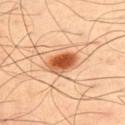lighting: cross-polarized
site: back
automated_metrics:
  area_mm2_approx: 8.5
  eccentricity: 0.75
  shape_asymmetry: 0.15
  cielab_L: 57
  cielab_a: 29
  cielab_b: 41
  vs_skin_darker_L: 17.0
  vs_skin_contrast_norm: 11.0
  border_irregularity_0_10: 2.0
  color_variation_0_10: 7.0
  peripheral_color_asymmetry: 2.0
  nevus_likeness_0_100: 100
  lesion_detection_confidence_0_100: 100
patient:
  sex: male
  age_approx: 50
image:
  source: total-body photography crop
  field_of_view_mm: 15